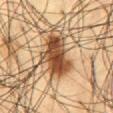* biopsy status · catalogued during a skin exam; not biopsied
* image · ~15 mm tile from a whole-body skin photo
* site · the abdomen
* patient · male, about 60 years old
* illumination · cross-polarized illumination